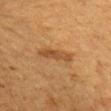This lesion was catalogued during total-body skin photography and was not selected for biopsy. The recorded lesion diameter is about 4 mm. From the left upper arm. A 15 mm crop from a total-body photograph taken for skin-cancer surveillance. A female patient about 40 years old. The tile uses cross-polarized illumination.